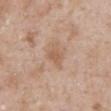Captured during whole-body skin photography for melanoma surveillance; the lesion was not biopsied. The lesion-visualizer software estimated a footprint of about 4 mm², an outline eccentricity of about 0.7 (0 = round, 1 = elongated), and a shape-asymmetry score of about 0.3 (0 = symmetric). It also reported a nevus-likeness score of about 0/100 and a lesion-detection confidence of about 100/100. The lesion's longest dimension is about 2.5 mm. The lesion is on the mid back. A male patient aged 48–52. A 15 mm crop from a total-body photograph taken for skin-cancer surveillance. Imaged with white-light lighting.